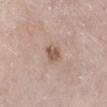The lesion was photographed on a routine skin check and not biopsied; there is no pathology result.
Longest diameter approximately 2.5 mm.
A lesion tile, about 15 mm wide, cut from a 3D total-body photograph.
The lesion is located on the right lower leg.
The patient is a male about 80 years old.